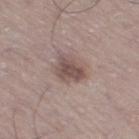Q: Was this lesion biopsied?
A: no biopsy performed (imaged during a skin exam)
Q: Who is the patient?
A: male, in their 70s
Q: Lesion location?
A: the left leg
Q: What is the imaging modality?
A: ~15 mm tile from a whole-body skin photo